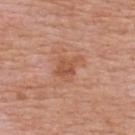biopsy status: catalogued during a skin exam; not biopsied
site: the upper back
tile lighting: white-light
subject: male, aged approximately 65
acquisition: 15 mm crop, total-body photography
diameter: about 3.5 mm